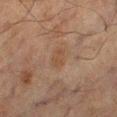  biopsy_status: not biopsied; imaged during a skin examination
  site: right lower leg
  image:
    source: total-body photography crop
    field_of_view_mm: 15
  patient:
    sex: male
    age_approx: 65
  automated_metrics:
    cielab_L: 38
    cielab_a: 15
    cielab_b: 26
    vs_skin_darker_L: 5.0
    vs_skin_contrast_norm: 5.5
    border_irregularity_0_10: 2.0
    color_variation_0_10: 2.0
    peripheral_color_asymmetry: 1.0
  lesion_size:
    long_diameter_mm_approx: 2.5